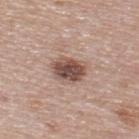No biopsy was performed on this lesion — it was imaged during a full skin examination and was not determined to be concerning. A roughly 15 mm field-of-view crop from a total-body skin photograph. The lesion is on the upper back. Longest diameter approximately 3.5 mm. The subject is a male aged approximately 55.